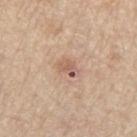Q: Is there a histopathology result?
A: total-body-photography surveillance lesion; no biopsy
Q: How large is the lesion?
A: ~2.5 mm (longest diameter)
Q: Who is the patient?
A: male, in their 70s
Q: What lighting was used for the tile?
A: white-light illumination
Q: What did automated image analysis measure?
A: a footprint of about 3.5 mm², an outline eccentricity of about 0.75 (0 = round, 1 = elongated), and a symmetry-axis asymmetry near 0.3; an average lesion color of about L≈59 a*≈20 b*≈28 (CIELAB), roughly 10 lightness units darker than nearby skin, and a normalized lesion–skin contrast near 6.5; border irregularity of about 2.5 on a 0–10 scale and a color-variation rating of about 6.5/10; a classifier nevus-likeness of about 0/100
Q: How was this image acquired?
A: ~15 mm tile from a whole-body skin photo
Q: Where on the body is the lesion?
A: the left thigh